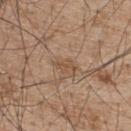Q: Is there a histopathology result?
A: no biopsy performed (imaged during a skin exam)
Q: What are the patient's age and sex?
A: male, in their mid- to late 50s
Q: What did automated image analysis measure?
A: a footprint of about 3 mm² and a shape-asymmetry score of about 0.4 (0 = symmetric); roughly 7 lightness units darker than nearby skin and a normalized border contrast of about 5.5; a within-lesion color-variation index near 0.5/10 and a peripheral color-asymmetry measure near 0; a classifier nevus-likeness of about 0/100 and a lesion-detection confidence of about 100/100
Q: How was this image acquired?
A: total-body-photography crop, ~15 mm field of view
Q: Lesion location?
A: the back
Q: How large is the lesion?
A: ≈2.5 mm
Q: What lighting was used for the tile?
A: white-light illumination